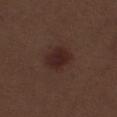Q: Was a biopsy performed?
A: no biopsy performed (imaged during a skin exam)
Q: Lesion size?
A: about 3.5 mm
Q: How was this image acquired?
A: ~15 mm tile from a whole-body skin photo
Q: How was the tile lit?
A: white-light
Q: What is the anatomic site?
A: the left thigh
Q: Patient demographics?
A: male, in their 70s